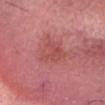biopsy status: no biopsy performed (imaged during a skin exam) | location: the head or neck | subject: male, aged approximately 70 | image source: ~15 mm tile from a whole-body skin photo.